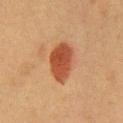Q: Was a biopsy performed?
A: catalogued during a skin exam; not biopsied
Q: How was the tile lit?
A: cross-polarized illumination
Q: Lesion size?
A: ~4.5 mm (longest diameter)
Q: What are the patient's age and sex?
A: female, in their 40s
Q: What is the anatomic site?
A: the abdomen
Q: What is the imaging modality?
A: ~15 mm crop, total-body skin-cancer survey